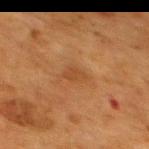Imaged during a routine full-body skin examination; the lesion was not biopsied and no histopathology is available.
About 2.5 mm across.
Imaged with cross-polarized lighting.
On the back.
A 15 mm crop from a total-body photograph taken for skin-cancer surveillance.
The subject is a female aged 53–57.